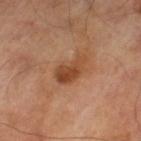Clinical impression:
The lesion was tiled from a total-body skin photograph and was not biopsied.
Background:
The recorded lesion diameter is about 4.5 mm. Imaged with cross-polarized lighting. Located on the left thigh. An algorithmic analysis of the crop reported a normalized border contrast of about 8. The patient is a male roughly 70 years of age. A roughly 15 mm field-of-view crop from a total-body skin photograph.